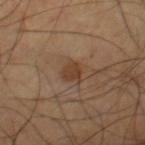* workup — catalogued during a skin exam; not biopsied
* illumination — cross-polarized
* automated metrics — a lesion area of about 4 mm², a shape eccentricity near 0.5, and a shape-asymmetry score of about 0.35 (0 = symmetric); a peripheral color-asymmetry measure near 0.5; a lesion-detection confidence of about 100/100
* acquisition — ~15 mm crop, total-body skin-cancer survey
* anatomic site — the right thigh
* lesion size — ~2.5 mm (longest diameter)
* patient — male, aged around 60